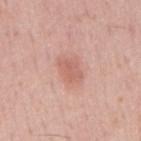Recorded during total-body skin imaging; not selected for excision or biopsy. This is a white-light tile. Measured at roughly 3.5 mm in maximum diameter. A lesion tile, about 15 mm wide, cut from a 3D total-body photograph. The lesion is on the mid back. A male patient aged 53 to 57.